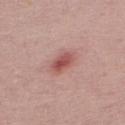workup = imaged on a skin check; not biopsied | image = total-body-photography crop, ~15 mm field of view | subject = male, aged around 30 | illumination = white-light illumination | diameter = about 3 mm.